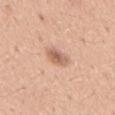The lesion was tiled from a total-body skin photograph and was not biopsied.
A close-up tile cropped from a whole-body skin photograph, about 15 mm across.
On the chest.
The patient is a male about 20 years old.